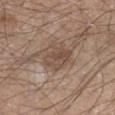Findings:
– notes: no biopsy performed (imaged during a skin exam)
– patient: male, in their mid- to late 40s
– TBP lesion metrics: a lesion color around L≈48 a*≈16 b*≈26 in CIELAB and about 8 CIELAB-L* units darker than the surrounding skin; a peripheral color-asymmetry measure near 1.5; a nevus-likeness score of about 0/100 and a lesion-detection confidence of about 100/100
– lighting: white-light
– lesion size: ≈4 mm
– anatomic site: the right lower leg
– imaging modality: ~15 mm crop, total-body skin-cancer survey